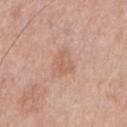{"biopsy_status": "not biopsied; imaged during a skin examination", "patient": {"sex": "male", "age_approx": 50}, "image": {"source": "total-body photography crop", "field_of_view_mm": 15}, "lighting": "white-light", "automated_metrics": {"nevus_likeness_0_100": 0}, "lesion_size": {"long_diameter_mm_approx": 3.5}, "site": "chest"}